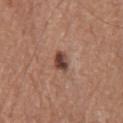Imaged during a routine full-body skin examination; the lesion was not biopsied and no histopathology is available. Cropped from a whole-body photographic skin survey; the tile spans about 15 mm. About 3 mm across. A male subject, aged 73 to 77. Automated image analysis of the tile measured an area of roughly 4.5 mm², a shape eccentricity near 0.75, and two-axis asymmetry of about 0.2. The analysis additionally found an average lesion color of about L≈43 a*≈21 b*≈25 (CIELAB), a lesion–skin lightness drop of about 14, and a normalized lesion–skin contrast near 10.5. And it measured a within-lesion color-variation index near 4.5/10 and peripheral color asymmetry of about 1.5. It also reported an automated nevus-likeness rating near 95 out of 100 and a detector confidence of about 100 out of 100 that the crop contains a lesion. Captured under white-light illumination. The lesion is located on the front of the torso.